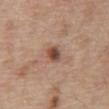Notes:
– notes: catalogued during a skin exam; not biopsied
– TBP lesion metrics: a lesion area of about 3.5 mm² and two-axis asymmetry of about 0.25; a lesion color around L≈47 a*≈20 b*≈28 in CIELAB and a normalized border contrast of about 9.5; border irregularity of about 2 on a 0–10 scale, a within-lesion color-variation index near 5/10, and a peripheral color-asymmetry measure near 1.5; a nevus-likeness score of about 90/100
– tile lighting: white-light
– location: the front of the torso
– patient: male, about 70 years old
– imaging modality: total-body-photography crop, ~15 mm field of view
– lesion diameter: about 2.5 mm